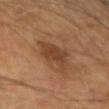Clinical summary:
This is a cross-polarized tile. Measured at roughly 4.5 mm in maximum diameter. Cropped from a whole-body photographic skin survey; the tile spans about 15 mm. The lesion is on the left forearm. The subject is a male about 70 years old.